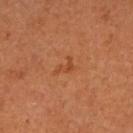Captured during whole-body skin photography for melanoma surveillance; the lesion was not biopsied. A lesion tile, about 15 mm wide, cut from a 3D total-body photograph. Located on the left arm. A female subject approximately 65 years of age.